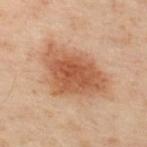Part of a total-body skin-imaging series; this lesion was reviewed on a skin check and was not flagged for biopsy. The patient is a male aged around 50. The lesion-visualizer software estimated a lesion area of about 25 mm², a shape eccentricity near 0.8, and two-axis asymmetry of about 0.3. The software also gave a lesion color around L≈45 a*≈20 b*≈29 in CIELAB, about 10 CIELAB-L* units darker than the surrounding skin, and a normalized lesion–skin contrast near 8.5. The software also gave a border-irregularity rating of about 3/10, a color-variation rating of about 4/10, and peripheral color asymmetry of about 1. On the upper back. Captured under cross-polarized illumination. Cropped from a total-body skin-imaging series; the visible field is about 15 mm. The recorded lesion diameter is about 7.5 mm.